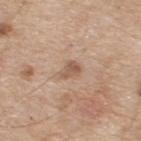Captured during whole-body skin photography for melanoma surveillance; the lesion was not biopsied.
A lesion tile, about 15 mm wide, cut from a 3D total-body photograph.
Longest diameter approximately 2.5 mm.
A male subject, about 70 years old.
From the upper back.